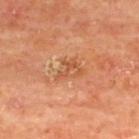{
  "biopsy_status": "not biopsied; imaged during a skin examination",
  "site": "upper back",
  "image": {
    "source": "total-body photography crop",
    "field_of_view_mm": 15
  },
  "patient": {
    "sex": "male",
    "age_approx": 65
  },
  "lesion_size": {
    "long_diameter_mm_approx": 3.0
  }
}